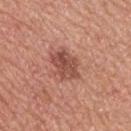{
  "biopsy_status": "not biopsied; imaged during a skin examination",
  "site": "upper back",
  "lesion_size": {
    "long_diameter_mm_approx": 4.0
  },
  "patient": {
    "sex": "male",
    "age_approx": 50
  },
  "lighting": "white-light",
  "image": {
    "source": "total-body photography crop",
    "field_of_view_mm": 15
  }
}